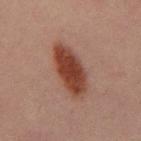This lesion was catalogued during total-body skin photography and was not selected for biopsy. A male patient, aged around 30. Cropped from a whole-body photographic skin survey; the tile spans about 15 mm. Automated image analysis of the tile measured a mean CIELAB color near L≈33 a*≈21 b*≈25, a lesion–skin lightness drop of about 13, and a lesion-to-skin contrast of about 12 (normalized; higher = more distinct). The analysis additionally found a border-irregularity index near 2/10, a within-lesion color-variation index near 3/10, and a peripheral color-asymmetry measure near 1. It also reported a nevus-likeness score of about 100/100. The lesion is on the mid back.